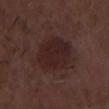Part of a total-body skin-imaging series; this lesion was reviewed on a skin check and was not flagged for biopsy.
About 5.5 mm across.
The tile uses white-light illumination.
A male patient roughly 60 years of age.
A close-up tile cropped from a whole-body skin photograph, about 15 mm across.
The lesion is located on the chest.